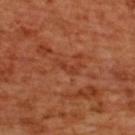Captured during whole-body skin photography for melanoma surveillance; the lesion was not biopsied. A region of skin cropped from a whole-body photographic capture, roughly 15 mm wide. This is a cross-polarized tile. Approximately 3 mm at its widest. Automated tile analysis of the lesion measured border irregularity of about 4 on a 0–10 scale and a peripheral color-asymmetry measure near 0. The lesion is on the upper back. A female patient, aged 53 to 57.